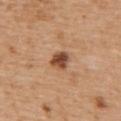Assessment: The lesion was photographed on a routine skin check and not biopsied; there is no pathology result. Acquisition and patient details: Longest diameter approximately 2.5 mm. The tile uses white-light illumination. A male subject, about 70 years old. A lesion tile, about 15 mm wide, cut from a 3D total-body photograph. The lesion is located on the upper back.